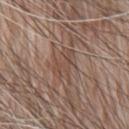Clinical impression: Captured during whole-body skin photography for melanoma surveillance; the lesion was not biopsied. Context: The lesion is located on the chest. This is a white-light tile. A male patient, aged 78 to 82. A lesion tile, about 15 mm wide, cut from a 3D total-body photograph. The lesion's longest dimension is about 4 mm.